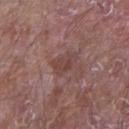Q: Was this lesion biopsied?
A: catalogued during a skin exam; not biopsied
Q: What kind of image is this?
A: ~15 mm crop, total-body skin-cancer survey
Q: Lesion location?
A: the right upper arm
Q: How was the tile lit?
A: white-light
Q: Who is the patient?
A: male, aged 63 to 67
Q: What is the lesion's diameter?
A: about 3 mm